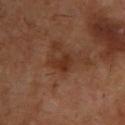Findings:
• notes — total-body-photography surveillance lesion; no biopsy
• image — 15 mm crop, total-body photography
• site — the upper back
• illumination — cross-polarized illumination
• size — ≈3 mm
• subject — male, about 55 years old